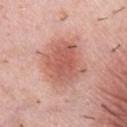notes: no biopsy performed (imaged during a skin exam)
image source: 15 mm crop, total-body photography
TBP lesion metrics: a lesion area of about 27 mm², an eccentricity of roughly 0.6, and a shape-asymmetry score of about 0.15 (0 = symmetric); internal color variation of about 4.5 on a 0–10 scale and radial color variation of about 1; an automated nevus-likeness rating near 95 out of 100 and a detector confidence of about 100 out of 100 that the crop contains a lesion
lighting: white-light
subject: male, aged 38 to 42
size: about 6.5 mm
location: the chest